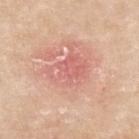Captured during whole-body skin photography for melanoma surveillance; the lesion was not biopsied.
The lesion is on the left forearm.
Measured at roughly 4.5 mm in maximum diameter.
A female subject in their 70s.
Imaged with white-light lighting.
A lesion tile, about 15 mm wide, cut from a 3D total-body photograph.